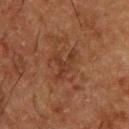Clinical impression: Part of a total-body skin-imaging series; this lesion was reviewed on a skin check and was not flagged for biopsy. Image and clinical context: The total-body-photography lesion software estimated a lesion area of about 6 mm² and a shape-asymmetry score of about 0.7 (0 = symmetric). It also reported a lesion color around L≈28 a*≈19 b*≈25 in CIELAB and about 5 CIELAB-L* units darker than the surrounding skin. The software also gave a border-irregularity index near 8.5/10 and a peripheral color-asymmetry measure near 0.5. It also reported lesion-presence confidence of about 95/100. The tile uses cross-polarized illumination. A male subject, aged around 50. The lesion is on the upper back. A 15 mm crop from a total-body photograph taken for skin-cancer surveillance.